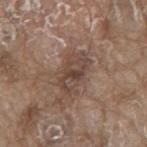Assessment: The lesion was photographed on a routine skin check and not biopsied; there is no pathology result. Context: Captured under white-light illumination. The lesion is located on the mid back. A close-up tile cropped from a whole-body skin photograph, about 15 mm across. A male patient approximately 80 years of age.